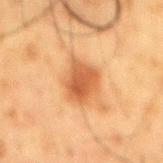workup: catalogued during a skin exam; not biopsied
illumination: cross-polarized illumination
image: ~15 mm crop, total-body skin-cancer survey
body site: the back
diameter: about 4 mm
patient: male, aged approximately 60